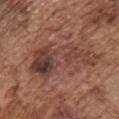Case summary:
– workup: imaged on a skin check; not biopsied
– automated metrics: an area of roughly 23 mm², an eccentricity of roughly 0.9, and two-axis asymmetry of about 0.55; border irregularity of about 9 on a 0–10 scale and radial color variation of about 2.5
– location: the chest
– subject: male, aged approximately 75
– image: 15 mm crop, total-body photography
– illumination: white-light